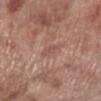Notes:
• notes · no biopsy performed (imaged during a skin exam)
• site · the right lower leg
• imaging modality · total-body-photography crop, ~15 mm field of view
• patient · male, roughly 70 years of age